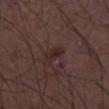image source: ~15 mm tile from a whole-body skin photo
subject: male, in their 50s
site: the right lower leg